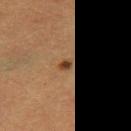Q: Was a biopsy performed?
A: no biopsy performed (imaged during a skin exam)
Q: What are the patient's age and sex?
A: female, about 40 years old
Q: How was this image acquired?
A: ~15 mm tile from a whole-body skin photo
Q: What is the anatomic site?
A: the right thigh